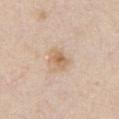<case>
  <biopsy_status>not biopsied; imaged during a skin examination</biopsy_status>
  <site>chest</site>
  <lighting>white-light</lighting>
  <patient>
    <sex>male</sex>
    <age_approx>35</age_approx>
  </patient>
  <automated_metrics>
    <cielab_L>64</cielab_L>
    <cielab_a>16</cielab_a>
    <cielab_b>33</cielab_b>
    <vs_skin_darker_L>9.0</vs_skin_darker_L>
    <border_irregularity_0_10>2.5</border_irregularity_0_10>
    <color_variation_0_10>3.5</color_variation_0_10>
    <peripheral_color_asymmetry>0.5</peripheral_color_asymmetry>
  </automated_metrics>
  <image>
    <source>total-body photography crop</source>
    <field_of_view_mm>15</field_of_view_mm>
  </image>
  <lesion_size>
    <long_diameter_mm_approx>3.0</long_diameter_mm_approx>
  </lesion_size>
</case>